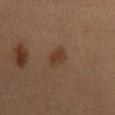lesion size=≈3 mm; acquisition=total-body-photography crop, ~15 mm field of view; patient=female, aged approximately 50; illumination=cross-polarized illumination.